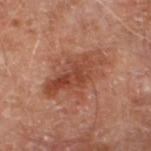notes: total-body-photography surveillance lesion; no biopsy | patient: male, approximately 70 years of age | size: about 7 mm | location: the left lower leg | image source: 15 mm crop, total-body photography.